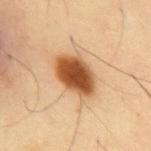Assessment:
Part of a total-body skin-imaging series; this lesion was reviewed on a skin check and was not flagged for biopsy.
Context:
A 15 mm crop from a total-body photograph taken for skin-cancer surveillance. Located on the abdomen. The recorded lesion diameter is about 5 mm. Captured under cross-polarized illumination. Automated image analysis of the tile measured a lesion area of about 13 mm² and an eccentricity of roughly 0.75. A male patient, aged around 55.